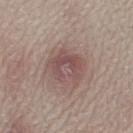{"lighting": "white-light", "patient": {"sex": "female", "age_approx": 60}, "lesion_size": {"long_diameter_mm_approx": 4.5}, "automated_metrics": {"area_mm2_approx": 12.0, "eccentricity": 0.6, "shape_asymmetry": 0.25, "cielab_L": 50, "cielab_a": 18, "cielab_b": 19, "vs_skin_darker_L": 9.0}, "site": "left lower leg", "image": {"source": "total-body photography crop", "field_of_view_mm": 15}}